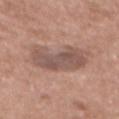– notes: catalogued during a skin exam; not biopsied
– location: the mid back
– diameter: ~6 mm (longest diameter)
– subject: female, aged approximately 75
– automated metrics: a footprint of about 17 mm²; a border-irregularity index near 4.5/10, a within-lesion color-variation index near 3/10, and a peripheral color-asymmetry measure near 1
– tile lighting: white-light
– acquisition: 15 mm crop, total-body photography